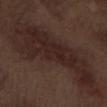Impression: No biopsy was performed on this lesion — it was imaged during a full skin examination and was not determined to be concerning. Acquisition and patient details: The lesion is on the left forearm. A 15 mm close-up tile from a total-body photography series done for melanoma screening. The subject is a male roughly 70 years of age.